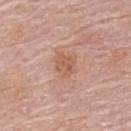notes — no biopsy performed (imaged during a skin exam); tile lighting — white-light illumination; site — the upper back; image source — ~15 mm tile from a whole-body skin photo; lesion diameter — about 3 mm; patient — female, approximately 65 years of age.